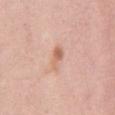Q: Was this lesion biopsied?
A: catalogued during a skin exam; not biopsied
Q: What are the patient's age and sex?
A: female, aged approximately 35
Q: What is the imaging modality?
A: 15 mm crop, total-body photography
Q: What is the anatomic site?
A: the chest